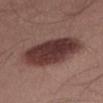The lesion was photographed on a routine skin check and not biopsied; there is no pathology result.
Captured under white-light illumination.
A close-up tile cropped from a whole-body skin photograph, about 15 mm across.
A male patient, approximately 55 years of age.
The total-body-photography lesion software estimated a footprint of about 28 mm², a shape eccentricity near 0.8, and two-axis asymmetry of about 0.15.
Approximately 8 mm at its widest.
On the abdomen.